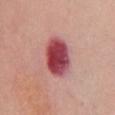No biopsy was performed on this lesion — it was imaged during a full skin examination and was not determined to be concerning. A roughly 15 mm field-of-view crop from a total-body skin photograph. The lesion's longest dimension is about 5.5 mm. The subject is a female aged approximately 75. The lesion is located on the front of the torso.